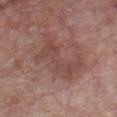follow-up — total-body-photography surveillance lesion; no biopsy
lighting — white-light illumination
acquisition — ~15 mm crop, total-body skin-cancer survey
patient — male, aged 63 to 67
automated metrics — a footprint of about 16 mm² and an eccentricity of roughly 0.9; a border-irregularity rating of about 8.5/10, a within-lesion color-variation index near 2.5/10, and radial color variation of about 1; an automated nevus-likeness rating near 0 out of 100 and a detector confidence of about 100 out of 100 that the crop contains a lesion
site — the front of the torso